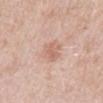Q: Is there a histopathology result?
A: catalogued during a skin exam; not biopsied
Q: What is the lesion's diameter?
A: about 3.5 mm
Q: Who is the patient?
A: male, approximately 50 years of age
Q: What is the imaging modality?
A: ~15 mm crop, total-body skin-cancer survey
Q: What lighting was used for the tile?
A: white-light illumination
Q: Where on the body is the lesion?
A: the mid back